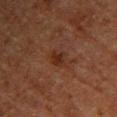biopsy status: imaged on a skin check; not biopsied | patient: female, aged 68 to 72 | anatomic site: the upper back | lesion size: ~2.5 mm (longest diameter) | illumination: cross-polarized illumination | automated metrics: a lesion area of about 4 mm², an outline eccentricity of about 0.7 (0 = round, 1 = elongated), and a shape-asymmetry score of about 0.25 (0 = symmetric); roughly 6 lightness units darker than nearby skin and a lesion-to-skin contrast of about 7.5 (normalized; higher = more distinct); an automated nevus-likeness rating near 10 out of 100 and a lesion-detection confidence of about 100/100 | acquisition: ~15 mm tile from a whole-body skin photo.